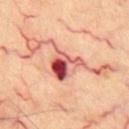| feature | finding |
|---|---|
| biopsy status | no biopsy performed (imaged during a skin exam) |
| site | the chest |
| lesion size | ~4.5 mm (longest diameter) |
| automated lesion analysis | a border-irregularity rating of about 5/10, a color-variation rating of about 10/10, and radial color variation of about 7.5; a nevus-likeness score of about 0/100 and a lesion-detection confidence of about 100/100 |
| image source | ~15 mm crop, total-body skin-cancer survey |
| patient | aged 63 to 67 |
| tile lighting | cross-polarized illumination |